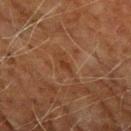Imaged during a routine full-body skin examination; the lesion was not biopsied and no histopathology is available. Longest diameter approximately 2.5 mm. A 15 mm crop from a total-body photograph taken for skin-cancer surveillance. The tile uses cross-polarized illumination. From the right upper arm. A male patient aged approximately 70.